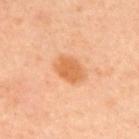notes: total-body-photography surveillance lesion; no biopsy
image: ~15 mm crop, total-body skin-cancer survey
anatomic site: the upper back
illumination: cross-polarized illumination
patient: male, in their 40s